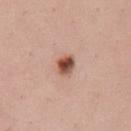– notes: total-body-photography surveillance lesion; no biopsy
– lighting: white-light illumination
– diameter: ≈3 mm
– subject: female, roughly 25 years of age
– location: the left upper arm
– image source: 15 mm crop, total-body photography
– automated metrics: a footprint of about 5 mm², a shape eccentricity near 0.7, and a shape-asymmetry score of about 0.2 (0 = symmetric); an average lesion color of about L≈52 a*≈23 b*≈29 (CIELAB), a lesion–skin lightness drop of about 17, and a lesion-to-skin contrast of about 11 (normalized; higher = more distinct)